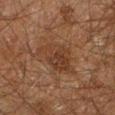Imaged during a routine full-body skin examination; the lesion was not biopsied and no histopathology is available.
A lesion tile, about 15 mm wide, cut from a 3D total-body photograph.
The lesion-visualizer software estimated an area of roughly 13 mm² and an eccentricity of roughly 0.7. The software also gave a lesion color around L≈28 a*≈16 b*≈24 in CIELAB, about 6 CIELAB-L* units darker than the surrounding skin, and a normalized border contrast of about 6.5. It also reported border irregularity of about 3.5 on a 0–10 scale and radial color variation of about 1.
A male patient, aged around 60.
Measured at roughly 5 mm in maximum diameter.
Imaged with cross-polarized lighting.
The lesion is located on the leg.